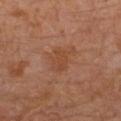Notes:
– follow-up — imaged on a skin check; not biopsied
– body site — the left leg
– tile lighting — cross-polarized illumination
– automated lesion analysis — a lesion area of about 5 mm², an eccentricity of roughly 0.75, and a symmetry-axis asymmetry near 0.25; an average lesion color of about L≈43 a*≈22 b*≈31 (CIELAB), about 6 CIELAB-L* units darker than the surrounding skin, and a normalized border contrast of about 5; border irregularity of about 2.5 on a 0–10 scale and a peripheral color-asymmetry measure near 1
– diameter — ≈3 mm
– patient — male, approximately 30 years of age
– imaging modality — 15 mm crop, total-body photography